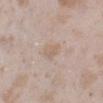biopsy status: total-body-photography surveillance lesion; no biopsy
lighting: white-light illumination
imaging modality: ~15 mm crop, total-body skin-cancer survey
automated metrics: an area of roughly 4.5 mm², a shape eccentricity near 0.7, and a symmetry-axis asymmetry near 0.35; a mean CIELAB color near L≈62 a*≈14 b*≈27, roughly 6 lightness units darker than nearby skin, and a normalized lesion–skin contrast near 5; border irregularity of about 3 on a 0–10 scale, internal color variation of about 1.5 on a 0–10 scale, and radial color variation of about 0.5
anatomic site: the left lower leg
diameter: ≈2.5 mm
subject: female, in their mid- to late 20s